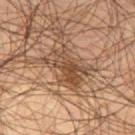• patient · male, in their mid- to late 50s
• tile lighting · cross-polarized illumination
• imaging modality · ~15 mm tile from a whole-body skin photo
• body site · the right thigh
• size · about 5.5 mm